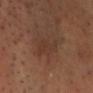Recorded during total-body skin imaging; not selected for excision or biopsy.
The total-body-photography lesion software estimated a classifier nevus-likeness of about 0/100 and a detector confidence of about 75 out of 100 that the crop contains a lesion.
The lesion is on the chest.
A male subject in their mid-50s.
A lesion tile, about 15 mm wide, cut from a 3D total-body photograph.